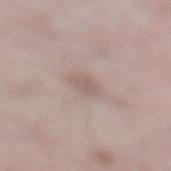workup — catalogued during a skin exam; not biopsied | patient — female, about 65 years old | acquisition — total-body-photography crop, ~15 mm field of view | location — the left lower leg.